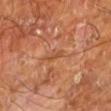This image is a 15 mm lesion crop taken from a total-body photograph. The tile uses cross-polarized illumination. About 2.5 mm across. The lesion is on the left lower leg. The subject is a male in their mid- to late 60s. The total-body-photography lesion software estimated an area of roughly 2 mm², an eccentricity of roughly 0.95, and a shape-asymmetry score of about 0.4 (0 = symmetric). The software also gave an automated nevus-likeness rating near 0 out of 100.